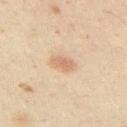Assessment: Captured during whole-body skin photography for melanoma surveillance; the lesion was not biopsied. Background: The subject is a male approximately 50 years of age. Imaged with cross-polarized lighting. A 15 mm close-up extracted from a 3D total-body photography capture. The lesion is on the right upper arm.